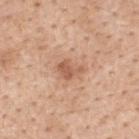Part of a total-body skin-imaging series; this lesion was reviewed on a skin check and was not flagged for biopsy. Imaged with white-light lighting. Approximately 3 mm at its widest. Automated tile analysis of the lesion measured an outline eccentricity of about 0.65 (0 = round, 1 = elongated) and two-axis asymmetry of about 0.3. The analysis additionally found an average lesion color of about L≈59 a*≈22 b*≈32 (CIELAB), a lesion–skin lightness drop of about 10, and a normalized border contrast of about 6.5. The software also gave border irregularity of about 3 on a 0–10 scale, a within-lesion color-variation index near 3.5/10, and a peripheral color-asymmetry measure near 1.5. The lesion is on the upper back. A female subject approximately 55 years of age. Cropped from a total-body skin-imaging series; the visible field is about 15 mm.